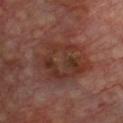The lesion was photographed on a routine skin check and not biopsied; there is no pathology result.
An algorithmic analysis of the crop reported a border-irregularity rating of about 4.5/10, a within-lesion color-variation index near 5.5/10, and a peripheral color-asymmetry measure near 1.5. The analysis additionally found an automated nevus-likeness rating near 0 out of 100.
From the front of the torso.
The subject is aged around 65.
A 15 mm crop from a total-body photograph taken for skin-cancer surveillance.
Longest diameter approximately 7 mm.